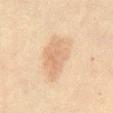Captured under cross-polarized illumination. From the abdomen. A male subject, about 60 years old. A 15 mm close-up extracted from a 3D total-body photography capture.